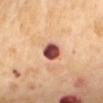Recorded during total-body skin imaging; not selected for excision or biopsy. Located on the mid back. Imaged with cross-polarized lighting. Cropped from a total-body skin-imaging series; the visible field is about 15 mm. A female subject roughly 55 years of age. The lesion's longest dimension is about 3 mm. The lesion-visualizer software estimated a footprint of about 6 mm². It also reported border irregularity of about 1.5 on a 0–10 scale and radial color variation of about 2.